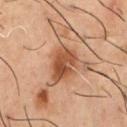biopsy status: imaged on a skin check; not biopsied
imaging modality: ~15 mm crop, total-body skin-cancer survey
automated lesion analysis: an area of roughly 11 mm², an outline eccentricity of about 0.75 (0 = round, 1 = elongated), and a shape-asymmetry score of about 0.2 (0 = symmetric); a border-irregularity rating of about 3/10, a color-variation rating of about 5.5/10, and radial color variation of about 2; a nevus-likeness score of about 85/100 and lesion-presence confidence of about 100/100
site: the front of the torso
patient: male, aged 53–57
illumination: cross-polarized illumination